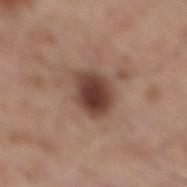{
  "biopsy_status": "not biopsied; imaged during a skin examination",
  "automated_metrics": {
    "cielab_L": 41,
    "cielab_a": 20,
    "cielab_b": 24,
    "vs_skin_contrast_norm": 11.0,
    "color_variation_0_10": 5.0,
    "peripheral_color_asymmetry": 1.5
  },
  "lighting": "white-light",
  "patient": {
    "sex": "male",
    "age_approx": 60
  },
  "lesion_size": {
    "long_diameter_mm_approx": 4.0
  },
  "image": {
    "source": "total-body photography crop",
    "field_of_view_mm": 15
  },
  "site": "mid back"
}